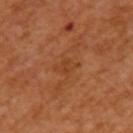follow-up = total-body-photography surveillance lesion; no biopsy
automated lesion analysis = an area of roughly 3.5 mm², an eccentricity of roughly 0.75, and two-axis asymmetry of about 0.2; a lesion color around L≈43 a*≈26 b*≈38 in CIELAB; internal color variation of about 1.5 on a 0–10 scale and radial color variation of about 0.5; a nevus-likeness score of about 0/100 and a detector confidence of about 100 out of 100 that the crop contains a lesion
acquisition = ~15 mm crop, total-body skin-cancer survey
patient = female, aged 53 to 57
anatomic site = the left upper arm
lighting = cross-polarized